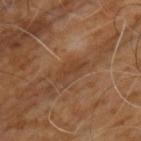The lesion was tiled from a total-body skin photograph and was not biopsied. On the chest. Cropped from a total-body skin-imaging series; the visible field is about 15 mm. A male subject, about 60 years old.